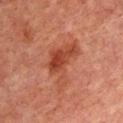Notes:
• workup — catalogued during a skin exam; not biopsied
• TBP lesion metrics — a mean CIELAB color near L≈36 a*≈25 b*≈28 and about 8 CIELAB-L* units darker than the surrounding skin; a border-irregularity index near 7/10, a color-variation rating of about 4/10, and radial color variation of about 1.5; a nevus-likeness score of about 15/100
• diameter — about 6 mm
• body site — the upper back
• illumination — cross-polarized
• image source — 15 mm crop, total-body photography
• patient — male, aged 63–67